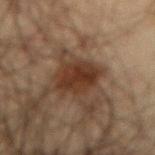<case>
  <biopsy_status>not biopsied; imaged during a skin examination</biopsy_status>
  <site>front of the torso</site>
  <image>
    <source>total-body photography crop</source>
    <field_of_view_mm>15</field_of_view_mm>
  </image>
  <patient>
    <sex>male</sex>
    <age_approx>65</age_approx>
  </patient>
</case>